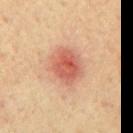biopsy status: catalogued during a skin exam; not biopsied | acquisition: ~15 mm tile from a whole-body skin photo | body site: the mid back | subject: male, aged approximately 65.